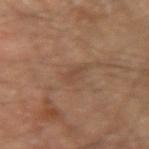Acquisition and patient details:
Cropped from a whole-body photographic skin survey; the tile spans about 15 mm. Located on the right upper arm. Automated image analysis of the tile measured a mean CIELAB color near L≈44 a*≈17 b*≈28 and a normalized border contrast of about 5. It also reported a classifier nevus-likeness of about 0/100. Approximately 3 mm at its widest. A male patient aged 63–67. The tile uses cross-polarized illumination.